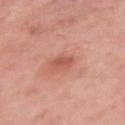{"biopsy_status": "not biopsied; imaged during a skin examination", "image": {"source": "total-body photography crop", "field_of_view_mm": 15}, "patient": {"sex": "female", "age_approx": 50}, "lesion_size": {"long_diameter_mm_approx": 2.5}, "site": "left upper arm"}